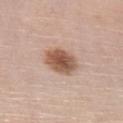Imaged during a routine full-body skin examination; the lesion was not biopsied and no histopathology is available. The lesion is on the chest. The subject is a female approximately 75 years of age. Automated tile analysis of the lesion measured a lesion area of about 10 mm². This is a white-light tile. Measured at roughly 4.5 mm in maximum diameter. A region of skin cropped from a whole-body photographic capture, roughly 15 mm wide.